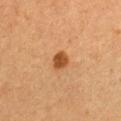<case>
  <lesion_size>
    <long_diameter_mm_approx>2.5</long_diameter_mm_approx>
  </lesion_size>
  <site>chest</site>
  <patient>
    <sex>female</sex>
    <age_approx>30</age_approx>
  </patient>
  <lighting>cross-polarized</lighting>
  <image>
    <source>total-body photography crop</source>
    <field_of_view_mm>15</field_of_view_mm>
  </image>
</case>